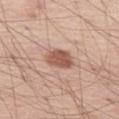The lesion was photographed on a routine skin check and not biopsied; there is no pathology result. Cropped from a total-body skin-imaging series; the visible field is about 15 mm. The patient is a male aged 53 to 57. Measured at roughly 4 mm in maximum diameter. From the left thigh.